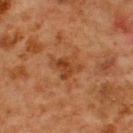Impression:
Imaged during a routine full-body skin examination; the lesion was not biopsied and no histopathology is available.
Clinical summary:
This image is a 15 mm lesion crop taken from a total-body photograph. The recorded lesion diameter is about 4 mm. From the back. A male patient aged approximately 65.